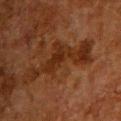workup: imaged on a skin check; not biopsied | imaging modality: ~15 mm crop, total-body skin-cancer survey | patient: male, aged 58–62 | body site: the front of the torso.